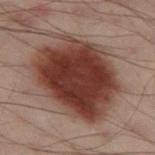tile lighting: cross-polarized | size: about 9 mm | acquisition: ~15 mm crop, total-body skin-cancer survey | automated lesion analysis: an area of roughly 50 mm² and a shape-asymmetry score of about 0.15 (0 = symmetric); a border-irregularity rating of about 2/10, a color-variation rating of about 5/10, and a peripheral color-asymmetry measure near 1.5 | body site: the left thigh | subject: male, about 50 years old.